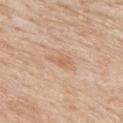  biopsy_status: not biopsied; imaged during a skin examination
  image:
    source: total-body photography crop
    field_of_view_mm: 15
  lighting: white-light
  site: upper back
  patient:
    sex: male
    age_approx: 85+